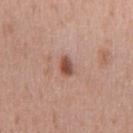Clinical impression: This lesion was catalogued during total-body skin photography and was not selected for biopsy. Acquisition and patient details: This image is a 15 mm lesion crop taken from a total-body photograph. Located on the chest. The patient is a male aged approximately 45.